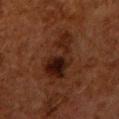Assessment:
This lesion was catalogued during total-body skin photography and was not selected for biopsy.
Acquisition and patient details:
An algorithmic analysis of the crop reported an automated nevus-likeness rating near 70 out of 100 and a detector confidence of about 100 out of 100 that the crop contains a lesion. A close-up tile cropped from a whole-body skin photograph, about 15 mm across. The patient is a female aged around 50. From the right upper arm. This is a cross-polarized tile. Measured at roughly 5.5 mm in maximum diameter.